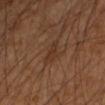biopsy status: total-body-photography surveillance lesion; no biopsy
body site: the left forearm
diameter: about 2.5 mm
imaging modality: 15 mm crop, total-body photography
subject: male, aged approximately 50
illumination: cross-polarized illumination